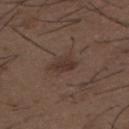Q: Was a biopsy performed?
A: catalogued during a skin exam; not biopsied
Q: Lesion location?
A: the back
Q: Lesion size?
A: ~3 mm (longest diameter)
Q: How was this image acquired?
A: 15 mm crop, total-body photography
Q: What are the patient's age and sex?
A: male, aged approximately 50